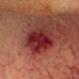Captured during whole-body skin photography for melanoma surveillance; the lesion was not biopsied. The subject is a male aged 33–37. The lesion is located on the head or neck. Cropped from a whole-body photographic skin survey; the tile spans about 15 mm.